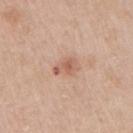{
  "biopsy_status": "not biopsied; imaged during a skin examination",
  "lighting": "white-light",
  "site": "right upper arm",
  "lesion_size": {
    "long_diameter_mm_approx": 2.5
  },
  "patient": {
    "sex": "male",
    "age_approx": 60
  },
  "image": {
    "source": "total-body photography crop",
    "field_of_view_mm": 15
  }
}